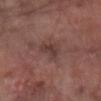Imaged during a routine full-body skin examination; the lesion was not biopsied and no histopathology is available.
A female subject, aged around 70.
The lesion is located on the right forearm.
A close-up tile cropped from a whole-body skin photograph, about 15 mm across.